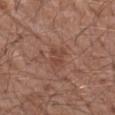No biopsy was performed on this lesion — it was imaged during a full skin examination and was not determined to be concerning. An algorithmic analysis of the crop reported an automated nevus-likeness rating near 0 out of 100 and a detector confidence of about 100 out of 100 that the crop contains a lesion. A male subject about 60 years old. This is a white-light tile. On the left forearm. About 3 mm across. A 15 mm close-up extracted from a 3D total-body photography capture.